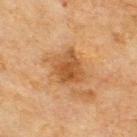| feature | finding |
|---|---|
| workup | catalogued during a skin exam; not biopsied |
| location | the upper back |
| lesion diameter | about 4 mm |
| acquisition | total-body-photography crop, ~15 mm field of view |
| tile lighting | cross-polarized |
| patient | male, aged approximately 70 |
| image-analysis metrics | a border-irregularity rating of about 2.5/10, internal color variation of about 3.5 on a 0–10 scale, and peripheral color asymmetry of about 1; an automated nevus-likeness rating near 30 out of 100 and a lesion-detection confidence of about 100/100 |